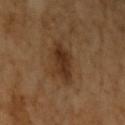biopsy_status: not biopsied; imaged during a skin examination
image:
  source: total-body photography crop
  field_of_view_mm: 15
patient:
  sex: female
  age_approx: 70
site: left upper arm